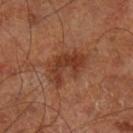{"biopsy_status": "not biopsied; imaged during a skin examination", "lighting": "cross-polarized", "site": "left lower leg", "image": {"source": "total-body photography crop", "field_of_view_mm": 15}, "patient": {"sex": "male", "age_approx": 70}, "lesion_size": {"long_diameter_mm_approx": 5.0}}